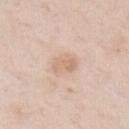Assessment: The lesion was tiled from a total-body skin photograph and was not biopsied. Image and clinical context: The tile uses white-light illumination. About 3.5 mm across. A 15 mm close-up extracted from a 3D total-body photography capture. The patient is a male aged approximately 50. From the chest.